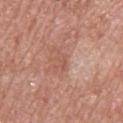biopsy_status: not biopsied; imaged during a skin examination
site: back
patient:
  sex: male
  age_approx: 60
image:
  source: total-body photography crop
  field_of_view_mm: 15
lighting: white-light
lesion_size:
  long_diameter_mm_approx: 2.5
automated_metrics:
  cielab_L: 55
  cielab_a: 24
  cielab_b: 29
  vs_skin_darker_L: 6.0
  vs_skin_contrast_norm: 5.0
  lesion_detection_confidence_0_100: 95